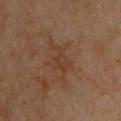Clinical impression:
The lesion was photographed on a routine skin check and not biopsied; there is no pathology result.
Background:
On the upper back. The total-body-photography lesion software estimated a lesion area of about 5.5 mm², a shape eccentricity near 0.8, and a shape-asymmetry score of about 0.45 (0 = symmetric). And it measured roughly 5 lightness units darker than nearby skin. The analysis additionally found a border-irregularity index near 6.5/10, a color-variation rating of about 2/10, and a peripheral color-asymmetry measure near 0.5. It also reported a nevus-likeness score of about 0/100 and lesion-presence confidence of about 100/100. A male subject approximately 55 years of age. Approximately 4 mm at its widest. Captured under cross-polarized illumination. A lesion tile, about 15 mm wide, cut from a 3D total-body photograph.